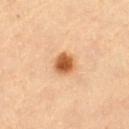Q: Was a biopsy performed?
A: imaged on a skin check; not biopsied
Q: Lesion location?
A: the left thigh
Q: Automated lesion metrics?
A: a footprint of about 5 mm² and a shape eccentricity near 0.5; a lesion color around L≈60 a*≈27 b*≈43 in CIELAB, roughly 18 lightness units darker than nearby skin, and a lesion-to-skin contrast of about 11.5 (normalized; higher = more distinct); border irregularity of about 1.5 on a 0–10 scale and a within-lesion color-variation index near 3.5/10; an automated nevus-likeness rating near 100 out of 100 and a lesion-detection confidence of about 100/100
Q: What kind of image is this?
A: 15 mm crop, total-body photography
Q: Patient demographics?
A: female, aged 68 to 72
Q: What lighting was used for the tile?
A: cross-polarized illumination
Q: Lesion size?
A: about 2.5 mm